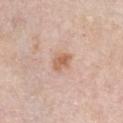Findings:
– notes · imaged on a skin check; not biopsied
– diameter · ≈2.5 mm
– automated metrics · about 10 CIELAB-L* units darker than the surrounding skin and a normalized border contrast of about 7.5; a border-irregularity index near 3/10, a within-lesion color-variation index near 3.5/10, and a peripheral color-asymmetry measure near 1; a nevus-likeness score of about 65/100 and a detector confidence of about 100 out of 100 that the crop contains a lesion
– anatomic site · the front of the torso
– imaging modality · ~15 mm tile from a whole-body skin photo
– subject · female, in their mid- to late 60s